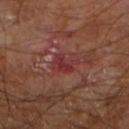Q: Is there a histopathology result?
A: no biopsy performed (imaged during a skin exam)
Q: Automated lesion metrics?
A: a footprint of about 5 mm² and a shape-asymmetry score of about 0.35 (0 = symmetric); an automated nevus-likeness rating near 0 out of 100
Q: What is the lesion's diameter?
A: ~3 mm (longest diameter)
Q: What is the anatomic site?
A: the right leg
Q: Who is the patient?
A: male, aged 58 to 62
Q: Illumination type?
A: cross-polarized illumination
Q: What is the imaging modality?
A: ~15 mm tile from a whole-body skin photo